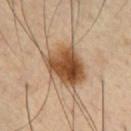Recorded during total-body skin imaging; not selected for excision or biopsy.